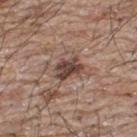Assessment: This lesion was catalogued during total-body skin photography and was not selected for biopsy. Acquisition and patient details: The tile uses white-light illumination. The recorded lesion diameter is about 3.5 mm. A 15 mm crop from a total-body photograph taken for skin-cancer surveillance. A male subject, about 65 years old. The total-body-photography lesion software estimated a shape eccentricity near 0.7 and a shape-asymmetry score of about 0.2 (0 = symmetric). The analysis additionally found a nevus-likeness score of about 0/100 and a lesion-detection confidence of about 100/100. The lesion is on the back.